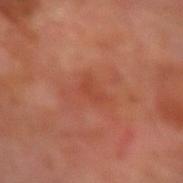Captured during whole-body skin photography for melanoma surveillance; the lesion was not biopsied. Longest diameter approximately 2.5 mm. A male patient approximately 70 years of age. A roughly 15 mm field-of-view crop from a total-body skin photograph. Located on the right forearm. Imaged with cross-polarized lighting. Automated image analysis of the tile measured a lesion area of about 3.5 mm² and a shape-asymmetry score of about 0.4 (0 = symmetric). The software also gave a lesion color around L≈45 a*≈29 b*≈33 in CIELAB and a normalized border contrast of about 4.5. The analysis additionally found internal color variation of about 0.5 on a 0–10 scale and radial color variation of about 0. The analysis additionally found an automated nevus-likeness rating near 0 out of 100 and lesion-presence confidence of about 100/100.